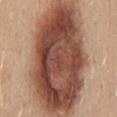• workup — total-body-photography surveillance lesion; no biopsy
• image — ~15 mm crop, total-body skin-cancer survey
• lesion diameter — ~17 mm (longest diameter)
• illumination — white-light illumination
• automated metrics — an average lesion color of about L≈49 a*≈22 b*≈28 (CIELAB)
• anatomic site — the mid back
• subject — female, aged around 45